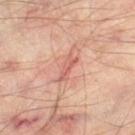The lesion was photographed on a routine skin check and not biopsied; there is no pathology result. The lesion-visualizer software estimated a lesion color around L≈60 a*≈27 b*≈28 in CIELAB, a lesion–skin lightness drop of about 9, and a normalized lesion–skin contrast near 6. The software also gave a border-irregularity index near 4.5/10, internal color variation of about 0 on a 0–10 scale, and radial color variation of about 0. From the left thigh. The patient is roughly 55 years of age. A roughly 15 mm field-of-view crop from a total-body skin photograph. The recorded lesion diameter is about 3.5 mm. The tile uses cross-polarized illumination.